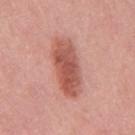  biopsy_status: not biopsied; imaged during a skin examination